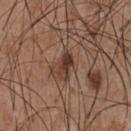Imaged during a routine full-body skin examination; the lesion was not biopsied and no histopathology is available.
The patient is a male roughly 55 years of age.
The tile uses white-light illumination.
On the front of the torso.
Cropped from a total-body skin-imaging series; the visible field is about 15 mm.